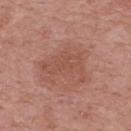workup: no biopsy performed (imaged during a skin exam) | patient: male, aged approximately 65 | image-analysis metrics: a footprint of about 13 mm², an outline eccentricity of about 0.8 (0 = round, 1 = elongated), and two-axis asymmetry of about 0.4; a lesion color around L≈51 a*≈24 b*≈28 in CIELAB and a lesion–skin lightness drop of about 6; a border-irregularity index near 5.5/10, a within-lesion color-variation index near 2/10, and peripheral color asymmetry of about 0.5; a classifier nevus-likeness of about 5/100 and lesion-presence confidence of about 100/100 | diameter: ~5.5 mm (longest diameter) | tile lighting: white-light | anatomic site: the upper back | image: total-body-photography crop, ~15 mm field of view.